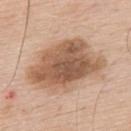Case summary:
– follow-up: no biopsy performed (imaged during a skin exam)
– patient: male, aged approximately 60
– acquisition: total-body-photography crop, ~15 mm field of view
– illumination: white-light illumination
– TBP lesion metrics: a shape eccentricity near 0.75 and a shape-asymmetry score of about 0.3 (0 = symmetric); a lesion color around L≈57 a*≈19 b*≈31 in CIELAB and roughly 14 lightness units darker than nearby skin; a border-irregularity rating of about 3/10, a color-variation rating of about 5.5/10, and peripheral color asymmetry of about 2
– location: the upper back